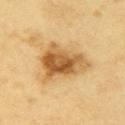biopsy status = imaged on a skin check; not biopsied | site = the right upper arm | imaging modality = 15 mm crop, total-body photography | patient = male, aged 58–62.